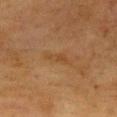| key | value |
|---|---|
| biopsy status | imaged on a skin check; not biopsied |
| site | the chest |
| image source | 15 mm crop, total-body photography |
| image-analysis metrics | a footprint of about 3 mm²; an average lesion color of about L≈37 a*≈17 b*≈31 (CIELAB) and a lesion–skin lightness drop of about 5; a within-lesion color-variation index near 0/10 and radial color variation of about 0; a classifier nevus-likeness of about 0/100 and a lesion-detection confidence of about 100/100 |
| subject | female, approximately 55 years of age |